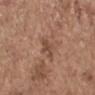{
  "lighting": "white-light",
  "patient": {
    "sex": "female",
    "age_approx": 65
  },
  "site": "left forearm",
  "image": {
    "source": "total-body photography crop",
    "field_of_view_mm": 15
  },
  "lesion_size": {
    "long_diameter_mm_approx": 3.0
  }
}